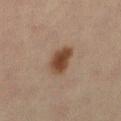<tbp_lesion>
<biopsy_status>not biopsied; imaged during a skin examination</biopsy_status>
<image>
  <source>total-body photography crop</source>
  <field_of_view_mm>15</field_of_view_mm>
</image>
<patient>
  <sex>female</sex>
  <age_approx>45</age_approx>
</patient>
<site>leg</site>
</tbp_lesion>